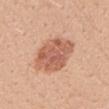Assessment: The lesion was tiled from a total-body skin photograph and was not biopsied. Image and clinical context: This is a white-light tile. A lesion tile, about 15 mm wide, cut from a 3D total-body photograph. On the right upper arm. The recorded lesion diameter is about 5 mm. A female patient, approximately 25 years of age.